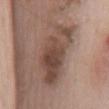Case summary:
* notes: no biopsy performed (imaged during a skin exam)
* patient: male, approximately 65 years of age
* body site: the mid back
* image source: ~15 mm tile from a whole-body skin photo